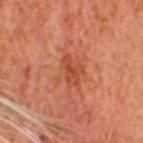Assessment: This lesion was catalogued during total-body skin photography and was not selected for biopsy. Clinical summary: A 15 mm close-up tile from a total-body photography series done for melanoma screening. The recorded lesion diameter is about 3.5 mm. The subject is a male roughly 65 years of age.